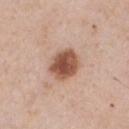<lesion>
<biopsy_status>not biopsied; imaged during a skin examination</biopsy_status>
<site>chest</site>
<image>
  <source>total-body photography crop</source>
  <field_of_view_mm>15</field_of_view_mm>
</image>
<automated_metrics>
  <area_mm2_approx>10.0</area_mm2_approx>
  <shape_asymmetry>0.15</shape_asymmetry>
  <cielab_L>53</cielab_L>
  <cielab_a>23</cielab_a>
  <cielab_b>30</cielab_b>
  <vs_skin_darker_L>16.0</vs_skin_darker_L>
  <vs_skin_contrast_norm>11.0</vs_skin_contrast_norm>
  <lesion_detection_confidence_0_100>100</lesion_detection_confidence_0_100>
</automated_metrics>
<patient>
  <sex>male</sex>
  <age_approx>60</age_approx>
</patient>
<lesion_size>
  <long_diameter_mm_approx>4.0</long_diameter_mm_approx>
</lesion_size>
<lighting>white-light</lighting>
</lesion>